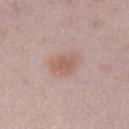The lesion was tiled from a total-body skin photograph and was not biopsied.
The lesion is located on the left lower leg.
This is a white-light tile.
A 15 mm close-up tile from a total-body photography series done for melanoma screening.
Approximately 3 mm at its widest.
Automated image analysis of the tile measured an eccentricity of roughly 0.75. And it measured roughly 8 lightness units darker than nearby skin and a lesion-to-skin contrast of about 6.5 (normalized; higher = more distinct). The software also gave a border-irregularity rating of about 1.5/10 and a color-variation rating of about 1.5/10.
A female patient roughly 30 years of age.